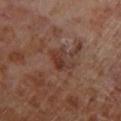workup: total-body-photography surveillance lesion; no biopsy
lesion diameter: ≈3.5 mm
subject: male, in their 70s
location: the left lower leg
TBP lesion metrics: an area of roughly 6 mm² and a shape eccentricity near 0.7; a classifier nevus-likeness of about 0/100 and lesion-presence confidence of about 100/100
acquisition: ~15 mm crop, total-body skin-cancer survey
tile lighting: cross-polarized